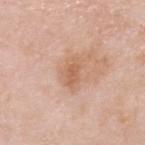notes: catalogued during a skin exam; not biopsied | image: ~15 mm tile from a whole-body skin photo | tile lighting: white-light illumination | patient: male, roughly 80 years of age | body site: the head or neck.